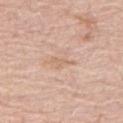Captured during whole-body skin photography for melanoma surveillance; the lesion was not biopsied.
About 3 mm across.
On the right thigh.
A female patient approximately 70 years of age.
Cropped from a total-body skin-imaging series; the visible field is about 15 mm.
Captured under white-light illumination.